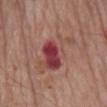{"biopsy_status": "not biopsied; imaged during a skin examination", "image": {"source": "total-body photography crop", "field_of_view_mm": 15}, "patient": {"sex": "male", "age_approx": 80}, "site": "mid back", "automated_metrics": {"area_mm2_approx": 17.0, "eccentricity": 0.8, "shape_asymmetry": 0.4, "cielab_L": 45, "cielab_a": 27, "cielab_b": 23, "vs_skin_darker_L": 10.0, "vs_skin_contrast_norm": 8.0, "border_irregularity_0_10": 6.0, "peripheral_color_asymmetry": 4.0}, "lesion_size": {"long_diameter_mm_approx": 6.5}, "lighting": "white-light"}